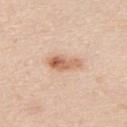{
  "biopsy_status": "not biopsied; imaged during a skin examination",
  "lesion_size": {
    "long_diameter_mm_approx": 4.0
  },
  "image": {
    "source": "total-body photography crop",
    "field_of_view_mm": 15
  },
  "site": "upper back",
  "patient": {
    "sex": "male",
    "age_approx": 40
  }
}